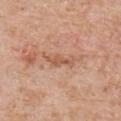Recorded during total-body skin imaging; not selected for excision or biopsy. A roughly 15 mm field-of-view crop from a total-body skin photograph. On the right upper arm. A male patient roughly 60 years of age.